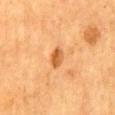Impression:
No biopsy was performed on this lesion — it was imaged during a full skin examination and was not determined to be concerning.
Image and clinical context:
The lesion is on the mid back. The patient is a female in their mid-50s. This is a cross-polarized tile. A 15 mm close-up extracted from a 3D total-body photography capture.